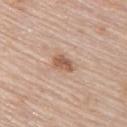workup: total-body-photography surveillance lesion; no biopsy | image-analysis metrics: a color-variation rating of about 3/10 and a peripheral color-asymmetry measure near 1; a nevus-likeness score of about 85/100 and a detector confidence of about 100 out of 100 that the crop contains a lesion | illumination: white-light illumination | acquisition: total-body-photography crop, ~15 mm field of view | body site: the back | size: ~3 mm (longest diameter) | patient: female, in their mid-60s.